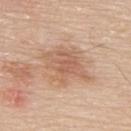Case summary:
* biopsy status — total-body-photography surveillance lesion; no biopsy
* lesion size — about 5 mm
* acquisition — 15 mm crop, total-body photography
* patient — male, aged 68–72
* illumination — white-light
* site — the upper back